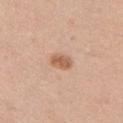workup=catalogued during a skin exam; not biopsied.